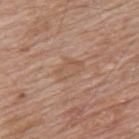notes: total-body-photography surveillance lesion; no biopsy
anatomic site: the back
image: 15 mm crop, total-body photography
patient: male, aged approximately 80